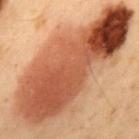Clinical impression:
Imaged during a routine full-body skin examination; the lesion was not biopsied and no histopathology is available.
Background:
Measured at roughly 18 mm in maximum diameter. Imaged with cross-polarized lighting. The lesion is located on the mid back. A male patient roughly 55 years of age. Cropped from a total-body skin-imaging series; the visible field is about 15 mm.